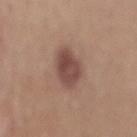Recorded during total-body skin imaging; not selected for excision or biopsy. Automated tile analysis of the lesion measured a footprint of about 12 mm² and an outline eccentricity of about 0.7 (0 = round, 1 = elongated). And it measured an average lesion color of about L≈47 a*≈20 b*≈23 (CIELAB), about 11 CIELAB-L* units darker than the surrounding skin, and a lesion-to-skin contrast of about 8.5 (normalized; higher = more distinct). Imaged with white-light lighting. From the mid back. Cropped from a whole-body photographic skin survey; the tile spans about 15 mm. The lesion's longest dimension is about 4.5 mm. The patient is a male approximately 50 years of age.